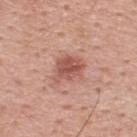No biopsy was performed on this lesion — it was imaged during a full skin examination and was not determined to be concerning. A male patient aged 53 to 57. This image is a 15 mm lesion crop taken from a total-body photograph. The lesion's longest dimension is about 4 mm. An algorithmic analysis of the crop reported a mean CIELAB color near L≈54 a*≈25 b*≈28, roughly 11 lightness units darker than nearby skin, and a normalized border contrast of about 7.5. It also reported a classifier nevus-likeness of about 50/100 and a detector confidence of about 100 out of 100 that the crop contains a lesion. On the upper back.